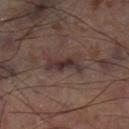Clinical impression:
The lesion was photographed on a routine skin check and not biopsied; there is no pathology result.
Background:
The lesion's longest dimension is about 4.5 mm. A roughly 15 mm field-of-view crop from a total-body skin photograph. The lesion-visualizer software estimated a lesion area of about 6 mm², an outline eccentricity of about 0.9 (0 = round, 1 = elongated), and a symmetry-axis asymmetry near 0.45. The software also gave an average lesion color of about L≈32 a*≈15 b*≈16 (CIELAB), a lesion–skin lightness drop of about 9, and a normalized border contrast of about 9.5. On the leg. Imaged with cross-polarized lighting.